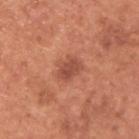follow-up = total-body-photography surveillance lesion; no biopsy
tile lighting = white-light illumination
patient = male, aged 63–67
lesion diameter = ~3 mm (longest diameter)
image = ~15 mm crop, total-body skin-cancer survey
anatomic site = the upper back